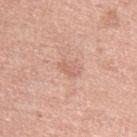Q: Is there a histopathology result?
A: catalogued during a skin exam; not biopsied
Q: What kind of image is this?
A: 15 mm crop, total-body photography
Q: Lesion location?
A: the left upper arm
Q: Who is the patient?
A: male, aged around 60
Q: What did automated image analysis measure?
A: an outline eccentricity of about 0.85 (0 = round, 1 = elongated) and a symmetry-axis asymmetry near 0.25; a border-irregularity index near 2.5/10; a detector confidence of about 100 out of 100 that the crop contains a lesion
Q: What lighting was used for the tile?
A: white-light
Q: How large is the lesion?
A: about 2.5 mm